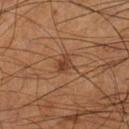{
  "biopsy_status": "not biopsied; imaged during a skin examination",
  "lesion_size": {
    "long_diameter_mm_approx": 2.5
  },
  "lighting": "cross-polarized",
  "patient": {
    "sex": "male",
    "age_approx": 55
  },
  "site": "left lower leg",
  "image": {
    "source": "total-body photography crop",
    "field_of_view_mm": 15
  }
}